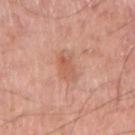• follow-up · imaged on a skin check; not biopsied
• lesion diameter · ~3.5 mm (longest diameter)
• tile lighting · white-light illumination
• TBP lesion metrics · an area of roughly 5.5 mm², an outline eccentricity of about 0.8 (0 = round, 1 = elongated), and two-axis asymmetry of about 0.3; an average lesion color of about L≈59 a*≈25 b*≈32 (CIELAB), a lesion–skin lightness drop of about 8, and a normalized lesion–skin contrast near 5.5; a nevus-likeness score of about 10/100 and a detector confidence of about 100 out of 100 that the crop contains a lesion
• site · the right upper arm
• subject · male, aged around 60
• image · total-body-photography crop, ~15 mm field of view